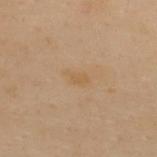follow-up — no biopsy performed (imaged during a skin exam) | image source — total-body-photography crop, ~15 mm field of view | site — the upper back | patient — female, aged 58–62 | lesion size — ≈2.5 mm.